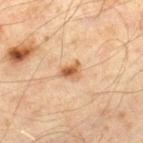notes: catalogued during a skin exam; not biopsied | location: the right lower leg | lesion diameter: about 2.5 mm | image source: ~15 mm tile from a whole-body skin photo | subject: male, in their mid- to late 40s | lighting: cross-polarized illumination.